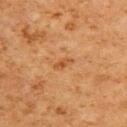follow-up: catalogued during a skin exam; not biopsied
diameter: ~2.5 mm (longest diameter)
image: ~15 mm tile from a whole-body skin photo
anatomic site: the upper back
patient: male, aged approximately 60
image-analysis metrics: a footprint of about 2.5 mm²; roughly 7 lightness units darker than nearby skin and a lesion-to-skin contrast of about 6 (normalized; higher = more distinct)